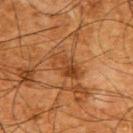Assessment: Captured during whole-body skin photography for melanoma surveillance; the lesion was not biopsied. Acquisition and patient details: From the upper back. The lesion-visualizer software estimated a lesion area of about 6.5 mm², an eccentricity of roughly 0.85, and a shape-asymmetry score of about 0.25 (0 = symmetric). The software also gave a within-lesion color-variation index near 6/10. The analysis additionally found a nevus-likeness score of about 10/100 and lesion-presence confidence of about 100/100. Imaged with cross-polarized lighting. A male subject roughly 65 years of age. A close-up tile cropped from a whole-body skin photograph, about 15 mm across.